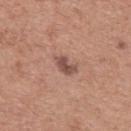– notes — total-body-photography surveillance lesion; no biopsy
– subject — female, about 60 years old
– size — about 3 mm
– anatomic site — the upper back
– imaging modality — 15 mm crop, total-body photography
– automated metrics — an area of roughly 4 mm² and two-axis asymmetry of about 0.3; a mean CIELAB color near L≈50 a*≈21 b*≈25, a lesion–skin lightness drop of about 11, and a lesion-to-skin contrast of about 8.5 (normalized; higher = more distinct); an automated nevus-likeness rating near 65 out of 100 and a lesion-detection confidence of about 100/100